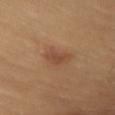Clinical impression: No biopsy was performed on this lesion — it was imaged during a full skin examination and was not determined to be concerning. Acquisition and patient details: The subject is a female aged 58–62. A roughly 15 mm field-of-view crop from a total-body skin photograph. The lesion is on the chest.